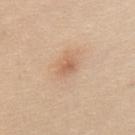biopsy status — imaged on a skin check; not biopsied
illumination — white-light illumination
location — the mid back
diameter — ~2 mm (longest diameter)
patient — female, aged approximately 20
imaging modality — total-body-photography crop, ~15 mm field of view